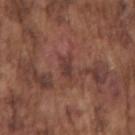A roughly 15 mm field-of-view crop from a total-body skin photograph. Approximately 2.5 mm at its widest. A male patient aged around 75. The lesion is located on the right upper arm.